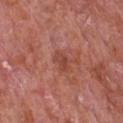| field | value |
|---|---|
| workup | no biopsy performed (imaged during a skin exam) |
| anatomic site | the chest |
| image source | total-body-photography crop, ~15 mm field of view |
| subject | male, approximately 65 years of age |